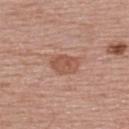{"biopsy_status": "not biopsied; imaged during a skin examination", "lighting": "white-light", "site": "upper back", "patient": {"sex": "female", "age_approx": 55}, "lesion_size": {"long_diameter_mm_approx": 3.5}, "automated_metrics": {"area_mm2_approx": 6.5, "eccentricity": 0.75, "cielab_L": 53, "cielab_a": 23, "cielab_b": 29, "vs_skin_darker_L": 9.0, "border_irregularity_0_10": 1.5, "color_variation_0_10": 2.5, "peripheral_color_asymmetry": 1.0, "nevus_likeness_0_100": 5, "lesion_detection_confidence_0_100": 100}, "image": {"source": "total-body photography crop", "field_of_view_mm": 15}}